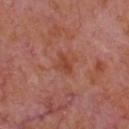anatomic site=the front of the torso; image=15 mm crop, total-body photography; subject=male, about 65 years old.